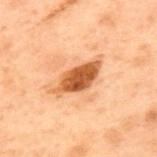TBP lesion metrics — a lesion area of about 11 mm² and a shape eccentricity near 0.9; a border-irregularity rating of about 3/10 and peripheral color asymmetry of about 1.5
lighting — cross-polarized
lesion diameter — ~5.5 mm (longest diameter)
image source — ~15 mm crop, total-body skin-cancer survey
subject — male, approximately 70 years of age
body site — the upper back